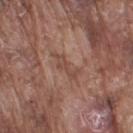Captured during whole-body skin photography for melanoma surveillance; the lesion was not biopsied. The tile uses white-light illumination. A 15 mm crop from a total-body photograph taken for skin-cancer surveillance. Automated tile analysis of the lesion measured a border-irregularity index near 6/10, a color-variation rating of about 1/10, and a peripheral color-asymmetry measure near 0. Measured at roughly 4 mm in maximum diameter. A male subject aged 73 to 77. From the right upper arm.